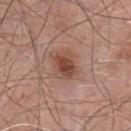Impression:
Recorded during total-body skin imaging; not selected for excision or biopsy.
Context:
A 15 mm close-up extracted from a 3D total-body photography capture. On the chest. Captured under white-light illumination. The patient is a male aged around 55.